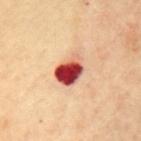No biopsy was performed on this lesion — it was imaged during a full skin examination and was not determined to be concerning.